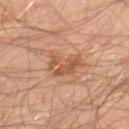Clinical impression: Part of a total-body skin-imaging series; this lesion was reviewed on a skin check and was not flagged for biopsy. Context: A male patient, aged 63 to 67. About 4.5 mm across. A close-up tile cropped from a whole-body skin photograph, about 15 mm across. The lesion is located on the left thigh. This is a cross-polarized tile.